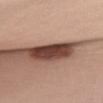Recorded during total-body skin imaging; not selected for excision or biopsy.
The lesion's longest dimension is about 7 mm.
From the chest.
The tile uses white-light illumination.
The patient is a female aged around 25.
A 15 mm close-up extracted from a 3D total-body photography capture.
Automated image analysis of the tile measured a lesion area of about 13 mm², a shape eccentricity near 0.95, and a symmetry-axis asymmetry near 0.2. The software also gave roughly 22 lightness units darker than nearby skin. The software also gave a border-irregularity rating of about 3.5/10, a within-lesion color-variation index near 4/10, and peripheral color asymmetry of about 1.5. The software also gave a lesion-detection confidence of about 45/100.